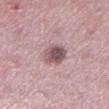The lesion was tiled from a total-body skin photograph and was not biopsied. A region of skin cropped from a whole-body photographic capture, roughly 15 mm wide. A female patient about 45 years old. Automated tile analysis of the lesion measured an area of roughly 6.5 mm², an eccentricity of roughly 0.6, and a symmetry-axis asymmetry near 0.15. And it measured a mean CIELAB color near L≈52 a*≈19 b*≈17, a lesion–skin lightness drop of about 15, and a lesion-to-skin contrast of about 9.5 (normalized; higher = more distinct). The software also gave a border-irregularity index near 1.5/10, a within-lesion color-variation index near 5.5/10, and radial color variation of about 1.5. And it measured a lesion-detection confidence of about 100/100. The lesion is located on the left lower leg. Longest diameter approximately 3 mm. Imaged with white-light lighting.